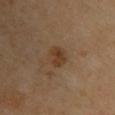{"biopsy_status": "not biopsied; imaged during a skin examination", "lighting": "cross-polarized", "patient": {"sex": "female", "age_approx": 70}, "site": "upper back", "image": {"source": "total-body photography crop", "field_of_view_mm": 15}}